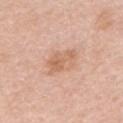The lesion was photographed on a routine skin check and not biopsied; there is no pathology result.
A female subject in their mid-40s.
From the left upper arm.
An algorithmic analysis of the crop reported a footprint of about 7.5 mm², an eccentricity of roughly 0.8, and a symmetry-axis asymmetry near 0.3. The software also gave a lesion color around L≈64 a*≈21 b*≈32 in CIELAB, roughly 9 lightness units darker than nearby skin, and a lesion-to-skin contrast of about 6 (normalized; higher = more distinct). The analysis additionally found a border-irregularity rating of about 3/10, internal color variation of about 4 on a 0–10 scale, and a peripheral color-asymmetry measure near 1. The software also gave a classifier nevus-likeness of about 5/100 and a detector confidence of about 100 out of 100 that the crop contains a lesion.
Cropped from a whole-body photographic skin survey; the tile spans about 15 mm.
This is a white-light tile.